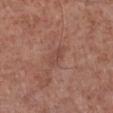workup: catalogued during a skin exam; not biopsied
subject: male, in their mid- to late 50s
size: ~2.5 mm (longest diameter)
acquisition: ~15 mm crop, total-body skin-cancer survey
tile lighting: white-light illumination
location: the right lower leg